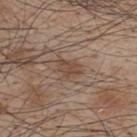Recorded during total-body skin imaging; not selected for excision or biopsy. Longest diameter approximately 2.5 mm. This is a white-light tile. A male subject, aged 43–47. The lesion is on the upper back. The lesion-visualizer software estimated an average lesion color of about L≈45 a*≈17 b*≈27 (CIELAB) and a normalized lesion–skin contrast near 6.5. A 15 mm crop from a total-body photograph taken for skin-cancer surveillance.